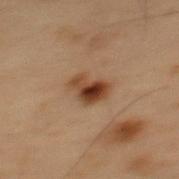biopsy status: no biopsy performed (imaged during a skin exam) | location: the mid back | tile lighting: cross-polarized | image source: 15 mm crop, total-body photography | size: ≈3.5 mm | patient: male, aged around 55 | image-analysis metrics: a mean CIELAB color near L≈33 a*≈17 b*≈27, a lesion–skin lightness drop of about 12, and a lesion-to-skin contrast of about 11 (normalized; higher = more distinct); border irregularity of about 3.5 on a 0–10 scale.